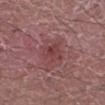Context:
Measured at roughly 2.5 mm in maximum diameter. Located on the left lower leg. Imaged with white-light lighting. Cropped from a total-body skin-imaging series; the visible field is about 15 mm. A male patient, aged approximately 45.